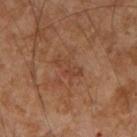Clinical impression: Recorded during total-body skin imaging; not selected for excision or biopsy. Context: Automated tile analysis of the lesion measured a border-irregularity rating of about 6.5/10, a within-lesion color-variation index near 0.5/10, and a peripheral color-asymmetry measure near 0. And it measured a detector confidence of about 100 out of 100 that the crop contains a lesion. A 15 mm close-up tile from a total-body photography series done for melanoma screening. Located on the right upper arm. Measured at roughly 4 mm in maximum diameter. A male patient, in their mid-50s. This is a cross-polarized tile.